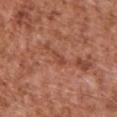A region of skin cropped from a whole-body photographic capture, roughly 15 mm wide. A male subject aged 73 to 77. The lesion is on the upper back. The lesion-visualizer software estimated a lesion–skin lightness drop of about 7 and a normalized lesion–skin contrast near 5.5. The analysis additionally found a border-irregularity index near 2.5/10, a color-variation rating of about 0.5/10, and a peripheral color-asymmetry measure near 0. Captured under white-light illumination.